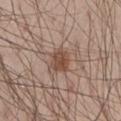Captured during whole-body skin photography for melanoma surveillance; the lesion was not biopsied. Imaged with white-light lighting. Located on the left thigh. An algorithmic analysis of the crop reported a peripheral color-asymmetry measure near 1. It also reported an automated nevus-likeness rating near 85 out of 100 and lesion-presence confidence of about 100/100. About 2.5 mm across. The patient is a male about 30 years old. A 15 mm close-up tile from a total-body photography series done for melanoma screening.